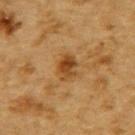| feature | finding |
|---|---|
| biopsy status | total-body-photography surveillance lesion; no biopsy |
| body site | the upper back |
| subject | male, in their mid-80s or older |
| lesion size | ≈3 mm |
| image | total-body-photography crop, ~15 mm field of view |
| lighting | cross-polarized |